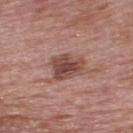Q: Is there a histopathology result?
A: imaged on a skin check; not biopsied
Q: Patient demographics?
A: male, aged 63–67
Q: What is the imaging modality?
A: total-body-photography crop, ~15 mm field of view
Q: How was the tile lit?
A: white-light illumination
Q: What did automated image analysis measure?
A: an area of roughly 9.5 mm², an eccentricity of roughly 0.55, and a shape-asymmetry score of about 0.3 (0 = symmetric); a normalized border contrast of about 9; border irregularity of about 3 on a 0–10 scale, internal color variation of about 4 on a 0–10 scale, and a peripheral color-asymmetry measure near 1.5
Q: How large is the lesion?
A: ~4 mm (longest diameter)
Q: Where on the body is the lesion?
A: the back